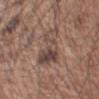Part of a total-body skin-imaging series; this lesion was reviewed on a skin check and was not flagged for biopsy.
This is a white-light tile.
From the left upper arm.
A male patient, roughly 55 years of age.
Cropped from a total-body skin-imaging series; the visible field is about 15 mm.